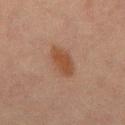Captured during whole-body skin photography for melanoma surveillance; the lesion was not biopsied.
A male patient, in their mid- to late 60s.
The lesion's longest dimension is about 4.5 mm.
A roughly 15 mm field-of-view crop from a total-body skin photograph.
Captured under cross-polarized illumination.
On the chest.
Automated tile analysis of the lesion measured about 7 CIELAB-L* units darker than the surrounding skin and a normalized lesion–skin contrast near 7.5. It also reported border irregularity of about 2 on a 0–10 scale, a color-variation rating of about 2/10, and peripheral color asymmetry of about 0.5. The software also gave lesion-presence confidence of about 100/100.